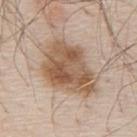– notes: catalogued during a skin exam; not biopsied
– patient: male, aged 78 to 82
– image source: 15 mm crop, total-body photography
– lesion diameter: ≈7.5 mm
– anatomic site: the upper back
– tile lighting: white-light illumination